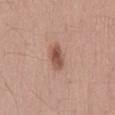This lesion was catalogued during total-body skin photography and was not selected for biopsy.
The lesion is on the back.
An algorithmic analysis of the crop reported an area of roughly 5.5 mm², an outline eccentricity of about 0.75 (0 = round, 1 = elongated), and a shape-asymmetry score of about 0.2 (0 = symmetric). The analysis additionally found a border-irregularity index near 2/10 and peripheral color asymmetry of about 1.5. The analysis additionally found an automated nevus-likeness rating near 95 out of 100 and a lesion-detection confidence of about 100/100.
A roughly 15 mm field-of-view crop from a total-body skin photograph.
This is a white-light tile.
The patient is a male aged 28–32.
The lesion's longest dimension is about 3 mm.